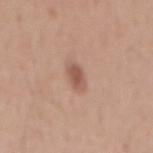Imaged during a routine full-body skin examination; the lesion was not biopsied and no histopathology is available.
Cropped from a total-body skin-imaging series; the visible field is about 15 mm.
Imaged with white-light lighting.
The recorded lesion diameter is about 3 mm.
A male patient, approximately 55 years of age.
The lesion-visualizer software estimated an average lesion color of about L≈55 a*≈21 b*≈27 (CIELAB), a lesion–skin lightness drop of about 10, and a lesion-to-skin contrast of about 7 (normalized; higher = more distinct).
The lesion is on the mid back.